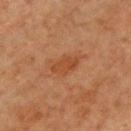<case>
  <patient>
    <sex>male</sex>
    <age_approx>65</age_approx>
  </patient>
  <image>
    <source>total-body photography crop</source>
    <field_of_view_mm>15</field_of_view_mm>
  </image>
  <site>front of the torso</site>
</case>